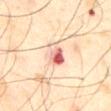Clinical impression: The lesion was photographed on a routine skin check and not biopsied; there is no pathology result. Acquisition and patient details: About 3.5 mm across. A male patient in their mid-40s. Cropped from a whole-body photographic skin survey; the tile spans about 15 mm. The lesion is located on the abdomen. Captured under cross-polarized illumination.